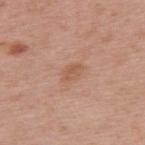<lesion>
<biopsy_status>not biopsied; imaged during a skin examination</biopsy_status>
<site>upper back</site>
<lighting>white-light</lighting>
<image>
  <source>total-body photography crop</source>
  <field_of_view_mm>15</field_of_view_mm>
</image>
<patient>
  <sex>female</sex>
  <age_approx>40</age_approx>
</patient>
</lesion>